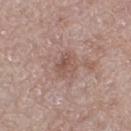Imaged during a routine full-body skin examination; the lesion was not biopsied and no histopathology is available. Cropped from a total-body skin-imaging series; the visible field is about 15 mm. The lesion's longest dimension is about 3 mm. The lesion is located on the right thigh. Automated tile analysis of the lesion measured an area of roughly 5 mm² and two-axis asymmetry of about 0.3. The analysis additionally found an average lesion color of about L≈50 a*≈12 b*≈24 (CIELAB), a lesion–skin lightness drop of about 8, and a normalized border contrast of about 6.5. The analysis additionally found a border-irregularity rating of about 2.5/10. This is a white-light tile. A female subject, roughly 60 years of age.